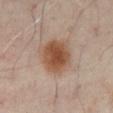The lesion was photographed on a routine skin check and not biopsied; there is no pathology result.
A male patient, aged 48–52.
From the abdomen.
The recorded lesion diameter is about 5 mm.
Automated tile analysis of the lesion measured about 12 CIELAB-L* units darker than the surrounding skin. It also reported a border-irregularity index near 1.5/10 and a within-lesion color-variation index near 4/10.
A region of skin cropped from a whole-body photographic capture, roughly 15 mm wide.